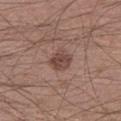{
  "biopsy_status": "not biopsied; imaged during a skin examination",
  "patient": {
    "sex": "male",
    "age_approx": 30
  },
  "site": "left lower leg",
  "image": {
    "source": "total-body photography crop",
    "field_of_view_mm": 15
  },
  "lighting": "white-light"
}